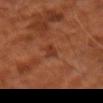Case summary:
• notes — no biopsy performed (imaged during a skin exam)
• image — ~15 mm tile from a whole-body skin photo
• tile lighting — cross-polarized
• size — about 2.5 mm
• anatomic site — the right upper arm
• patient — male, about 60 years old
• automated metrics — an average lesion color of about L≈33 a*≈25 b*≈30 (CIELAB), roughly 7 lightness units darker than nearby skin, and a lesion-to-skin contrast of about 6.5 (normalized; higher = more distinct)